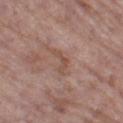Part of a total-body skin-imaging series; this lesion was reviewed on a skin check and was not flagged for biopsy. The subject is a female aged around 85 or older. The tile uses white-light illumination. Cropped from a whole-body photographic skin survey; the tile spans about 15 mm. Longest diameter approximately 3.5 mm. The lesion-visualizer software estimated an average lesion color of about L≈50 a*≈20 b*≈26 (CIELAB) and a lesion-to-skin contrast of about 6 (normalized; higher = more distinct). The software also gave an automated nevus-likeness rating near 0 out of 100 and a lesion-detection confidence of about 85/100. From the leg.